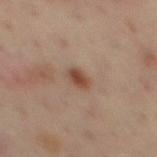| key | value |
|---|---|
| follow-up | total-body-photography surveillance lesion; no biopsy |
| image source | total-body-photography crop, ~15 mm field of view |
| patient | male, in their mid- to late 40s |
| lesion size | ~2.5 mm (longest diameter) |
| location | the mid back |
| tile lighting | cross-polarized illumination |